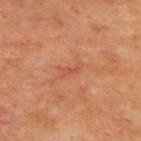Part of a total-body skin-imaging series; this lesion was reviewed on a skin check and was not flagged for biopsy. A region of skin cropped from a whole-body photographic capture, roughly 15 mm wide. The lesion is located on the upper back. Automated tile analysis of the lesion measured a nevus-likeness score of about 0/100. A subject in their mid- to late 60s. Captured under cross-polarized illumination.